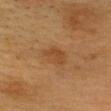Imaged during a routine full-body skin examination; the lesion was not biopsied and no histopathology is available. On the head or neck. This is a cross-polarized tile. A male patient, about 60 years old. About 2.5 mm across. A 15 mm close-up extracted from a 3D total-body photography capture.